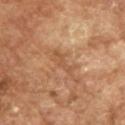Impression:
Imaged during a routine full-body skin examination; the lesion was not biopsied and no histopathology is available.
Clinical summary:
Approximately 4.5 mm at its widest. This is a cross-polarized tile. A male subject, about 65 years old. Automated image analysis of the tile measured about 7 CIELAB-L* units darker than the surrounding skin and a normalized border contrast of about 5. The analysis additionally found a border-irregularity index near 6/10, internal color variation of about 0 on a 0–10 scale, and a peripheral color-asymmetry measure near 0. A region of skin cropped from a whole-body photographic capture, roughly 15 mm wide.